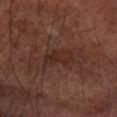{"biopsy_status": "not biopsied; imaged during a skin examination", "site": "right forearm", "patient": {"sex": "male", "age_approx": 65}, "image": {"source": "total-body photography crop", "field_of_view_mm": 15}}